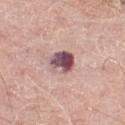biopsy status = total-body-photography surveillance lesion; no biopsy | tile lighting = white-light | lesion size = ≈3.5 mm | subject = male, aged 78 to 82 | body site = the leg | image = ~15 mm crop, total-body skin-cancer survey | image-analysis metrics = a lesion area of about 8 mm² and a symmetry-axis asymmetry near 0.2; an average lesion color of about L≈52 a*≈23 b*≈15 (CIELAB) and about 19 CIELAB-L* units darker than the surrounding skin; lesion-presence confidence of about 100/100.